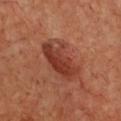follow-up = no biopsy performed (imaged during a skin exam) | lighting = cross-polarized | TBP lesion metrics = a lesion area of about 17 mm² and an eccentricity of roughly 0.8; a border-irregularity rating of about 3/10, a within-lesion color-variation index near 7/10, and a peripheral color-asymmetry measure near 2.5; a nevus-likeness score of about 65/100 and a detector confidence of about 100 out of 100 that the crop contains a lesion | size = ~6 mm (longest diameter) | subject = female, aged 43–47 | imaging modality = ~15 mm crop, total-body skin-cancer survey | site = the chest.